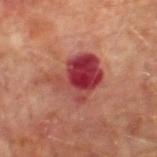<record>
  <biopsy_status>not biopsied; imaged during a skin examination</biopsy_status>
  <site>right lower leg</site>
  <automated_metrics>
    <cielab_L>42</cielab_L>
    <cielab_a>34</cielab_a>
    <cielab_b>25</cielab_b>
    <vs_skin_contrast_norm>11.0</vs_skin_contrast_norm>
    <nevus_likeness_0_100>0</nevus_likeness_0_100>
  </automated_metrics>
  <patient>
    <sex>male</sex>
    <age_approx>65</age_approx>
  </patient>
  <image>
    <source>total-body photography crop</source>
    <field_of_view_mm>15</field_of_view_mm>
  </image>
  <lighting>cross-polarized</lighting>
  <lesion_size>
    <long_diameter_mm_approx>5.5</long_diameter_mm_approx>
  </lesion_size>
</record>